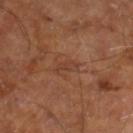Clinical impression: Imaged during a routine full-body skin examination; the lesion was not biopsied and no histopathology is available. Image and clinical context: The patient is a male aged approximately 60. The recorded lesion diameter is about 3 mm. A close-up tile cropped from a whole-body skin photograph, about 15 mm across. This is a cross-polarized tile. Located on the right leg.